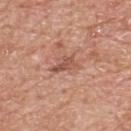Acquisition and patient details: The lesion is on the back. The patient is a male aged around 60. The tile uses white-light illumination. The lesion's longest dimension is about 3 mm. A roughly 15 mm field-of-view crop from a total-body skin photograph.